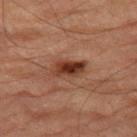Findings:
* imaging modality · total-body-photography crop, ~15 mm field of view
* body site · the right thigh
* lighting · cross-polarized
* subject · male, aged 83 or over
* diameter · ~4 mm (longest diameter)